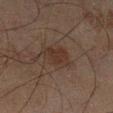notes: total-body-photography surveillance lesion; no biopsy
patient: male, aged 43 to 47
image: ~15 mm tile from a whole-body skin photo
lighting: cross-polarized illumination
location: the leg
diameter: ~4 mm (longest diameter)